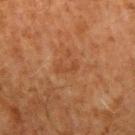Q: Is there a histopathology result?
A: no biopsy performed (imaged during a skin exam)
Q: Lesion location?
A: the left upper arm
Q: How was the tile lit?
A: cross-polarized illumination
Q: Lesion size?
A: ≈3 mm
Q: What are the patient's age and sex?
A: male, aged 68 to 72
Q: How was this image acquired?
A: ~15 mm tile from a whole-body skin photo
Q: Automated lesion metrics?
A: a footprint of about 3 mm² and a symmetry-axis asymmetry near 0.35; a lesion color around L≈38 a*≈21 b*≈32 in CIELAB, a lesion–skin lightness drop of about 5, and a lesion-to-skin contrast of about 4.5 (normalized; higher = more distinct); a nevus-likeness score of about 0/100